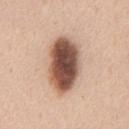{"biopsy_status": "not biopsied; imaged during a skin examination", "image": {"source": "total-body photography crop", "field_of_view_mm": 15}, "lesion_size": {"long_diameter_mm_approx": 7.5}, "site": "chest", "automated_metrics": {"area_mm2_approx": 23.0, "eccentricity": 0.85, "shape_asymmetry": 0.1, "border_irregularity_0_10": 1.5, "color_variation_0_10": 9.0, "peripheral_color_asymmetry": 3.0, "nevus_likeness_0_100": 100, "lesion_detection_confidence_0_100": 100}, "lighting": "white-light", "patient": {"sex": "male", "age_approx": 45}}